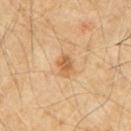workup = imaged on a skin check; not biopsied
body site = the chest
automated lesion analysis = an area of roughly 4.5 mm², an eccentricity of roughly 0.7, and two-axis asymmetry of about 0.2; a classifier nevus-likeness of about 35/100
image source = ~15 mm crop, total-body skin-cancer survey
illumination = cross-polarized illumination
lesion diameter = about 3 mm
subject = male, about 60 years old